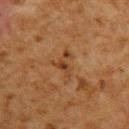Recorded during total-body skin imaging; not selected for excision or biopsy. A 15 mm close-up tile from a total-body photography series done for melanoma screening. Captured under cross-polarized illumination. An algorithmic analysis of the crop reported an area of roughly 5 mm², an eccentricity of roughly 0.8, and a shape-asymmetry score of about 0.65 (0 = symmetric). The analysis additionally found border irregularity of about 8 on a 0–10 scale, a color-variation rating of about 4/10, and peripheral color asymmetry of about 1. And it measured a lesion-detection confidence of about 85/100. From the upper back. About 3.5 mm across. The patient is a male aged around 60.